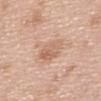biopsy status=no biopsy performed (imaged during a skin exam)
patient=female, approximately 50 years of age
diameter=≈4 mm
image source=~15 mm tile from a whole-body skin photo
site=the upper back